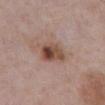Impression: Part of a total-body skin-imaging series; this lesion was reviewed on a skin check and was not flagged for biopsy. Acquisition and patient details: A male patient, in their mid-70s. An algorithmic analysis of the crop reported an area of roughly 9 mm² and an eccentricity of roughly 0.65. The software also gave a lesion color around L≈48 a*≈19 b*≈26 in CIELAB, roughly 12 lightness units darker than nearby skin, and a normalized border contrast of about 9.5. The analysis additionally found a border-irregularity rating of about 2/10 and peripheral color asymmetry of about 3.5. Located on the abdomen. A 15 mm close-up tile from a total-body photography series done for melanoma screening. Captured under white-light illumination.